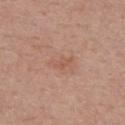The lesion was tiled from a total-body skin photograph and was not biopsied.
The lesion-visualizer software estimated a footprint of about 3 mm² and a shape eccentricity near 0.85. And it measured a lesion color around L≈55 a*≈22 b*≈29 in CIELAB, about 6 CIELAB-L* units darker than the surrounding skin, and a normalized lesion–skin contrast near 4.5. The software also gave a within-lesion color-variation index near 1/10 and peripheral color asymmetry of about 0.5. The analysis additionally found lesion-presence confidence of about 100/100.
The subject is a female about 50 years old.
Cropped from a total-body skin-imaging series; the visible field is about 15 mm.
The lesion is on the chest.
Approximately 2.5 mm at its widest.